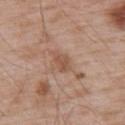Q: Was a biopsy performed?
A: total-body-photography surveillance lesion; no biopsy
Q: What lighting was used for the tile?
A: white-light illumination
Q: Lesion size?
A: ~2.5 mm (longest diameter)
Q: Patient demographics?
A: male, aged approximately 55
Q: Automated lesion metrics?
A: two-axis asymmetry of about 0.2; a border-irregularity index near 2/10, a within-lesion color-variation index near 2/10, and a peripheral color-asymmetry measure near 1
Q: What is the imaging modality?
A: 15 mm crop, total-body photography
Q: What is the anatomic site?
A: the upper back